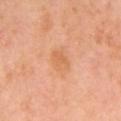No biopsy was performed on this lesion — it was imaged during a full skin examination and was not determined to be concerning.
The recorded lesion diameter is about 3 mm.
This image is a 15 mm lesion crop taken from a total-body photograph.
The total-body-photography lesion software estimated a lesion area of about 5 mm² and a symmetry-axis asymmetry near 0.2. It also reported a mean CIELAB color near L≈65 a*≈26 b*≈41, roughly 6 lightness units darker than nearby skin, and a normalized lesion–skin contrast near 5.
On the right upper arm.
The subject is a female about 55 years old.